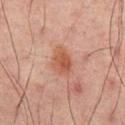Q: Was a biopsy performed?
A: imaged on a skin check; not biopsied
Q: Patient demographics?
A: male, aged around 65
Q: Where on the body is the lesion?
A: the mid back
Q: Automated lesion metrics?
A: an area of roughly 7.5 mm² and an eccentricity of roughly 0.7; a lesion color around L≈43 a*≈21 b*≈26 in CIELAB, roughly 8 lightness units darker than nearby skin, and a normalized lesion–skin contrast near 7.5; a border-irregularity index near 1.5/10 and internal color variation of about 3.5 on a 0–10 scale
Q: What kind of image is this?
A: ~15 mm crop, total-body skin-cancer survey